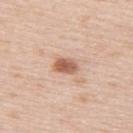The lesion was tiled from a total-body skin photograph and was not biopsied.
Measured at roughly 3 mm in maximum diameter.
A 15 mm crop from a total-body photograph taken for skin-cancer surveillance.
The patient is a female in their mid- to late 60s.
On the upper back.
Captured under white-light illumination.